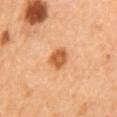Impression:
Recorded during total-body skin imaging; not selected for excision or biopsy.